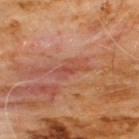notes: catalogued during a skin exam; not biopsied
image: ~15 mm crop, total-body skin-cancer survey
subject: male, aged approximately 60
anatomic site: the chest
illumination: cross-polarized illumination